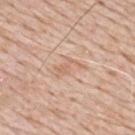Case summary:
– biopsy status — no biopsy performed (imaged during a skin exam)
– lighting — white-light illumination
– site — the mid back
– size — ~3.5 mm (longest diameter)
– automated metrics — a footprint of about 3.5 mm², an outline eccentricity of about 0.85 (0 = round, 1 = elongated), and two-axis asymmetry of about 0.55; a lesion color around L≈64 a*≈20 b*≈31 in CIELAB, a lesion–skin lightness drop of about 7, and a normalized lesion–skin contrast near 5; a border-irregularity rating of about 7.5/10, a color-variation rating of about 1/10, and peripheral color asymmetry of about 0; a classifier nevus-likeness of about 0/100 and lesion-presence confidence of about 100/100
– image source — 15 mm crop, total-body photography
– patient — male, approximately 80 years of age